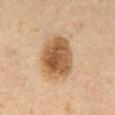Assessment: Captured during whole-body skin photography for melanoma surveillance; the lesion was not biopsied. Context: Measured at roughly 5.5 mm in maximum diameter. From the abdomen. A close-up tile cropped from a whole-body skin photograph, about 15 mm across. A male patient aged 58 to 62. Imaged with cross-polarized lighting. The total-body-photography lesion software estimated a lesion area of about 19 mm², a shape eccentricity near 0.65, and a symmetry-axis asymmetry near 0.15. It also reported a mean CIELAB color near L≈45 a*≈16 b*≈31, a lesion–skin lightness drop of about 13, and a lesion-to-skin contrast of about 10 (normalized; higher = more distinct). The analysis additionally found a color-variation rating of about 5/10 and a peripheral color-asymmetry measure near 1.5.